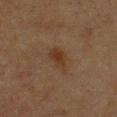Recorded during total-body skin imaging; not selected for excision or biopsy. The subject is a female aged 38 to 42. Longest diameter approximately 3.5 mm. A region of skin cropped from a whole-body photographic capture, roughly 15 mm wide. The lesion-visualizer software estimated border irregularity of about 2.5 on a 0–10 scale, internal color variation of about 2.5 on a 0–10 scale, and radial color variation of about 0.5. And it measured a nevus-likeness score of about 65/100 and lesion-presence confidence of about 100/100. Located on the chest.